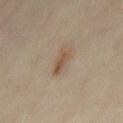Clinical summary: The tile uses cross-polarized illumination. A male subject, approximately 45 years of age. Located on the chest. A close-up tile cropped from a whole-body skin photograph, about 15 mm across.